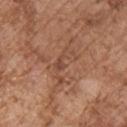No biopsy was performed on this lesion — it was imaged during a full skin examination and was not determined to be concerning. The subject is a male in their mid-70s. Captured under white-light illumination. A region of skin cropped from a whole-body photographic capture, roughly 15 mm wide. Located on the chest. The lesion-visualizer software estimated a footprint of about 6 mm², an outline eccentricity of about 0.9 (0 = round, 1 = elongated), and a shape-asymmetry score of about 0.6 (0 = symmetric). The analysis additionally found a classifier nevus-likeness of about 0/100 and lesion-presence confidence of about 70/100.